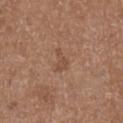Captured during whole-body skin photography for melanoma surveillance; the lesion was not biopsied. The lesion is on the right lower leg. Cropped from a whole-body photographic skin survey; the tile spans about 15 mm. A male subject, in their mid-70s. Measured at roughly 2.5 mm in maximum diameter. The tile uses white-light illumination.